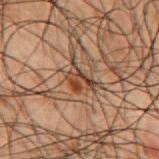Clinical impression:
Imaged during a routine full-body skin examination; the lesion was not biopsied and no histopathology is available.
Context:
A 15 mm crop from a total-body photograph taken for skin-cancer surveillance. The subject is a male aged 48–52. Imaged with cross-polarized lighting. The total-body-photography lesion software estimated a footprint of about 5 mm², a shape eccentricity near 0.7, and a symmetry-axis asymmetry near 0.4. It also reported a mean CIELAB color near L≈33 a*≈17 b*≈24, a lesion–skin lightness drop of about 9, and a lesion-to-skin contrast of about 8.5 (normalized; higher = more distinct). And it measured border irregularity of about 4 on a 0–10 scale. The software also gave an automated nevus-likeness rating near 0 out of 100 and a detector confidence of about 90 out of 100 that the crop contains a lesion. The lesion is on the back.